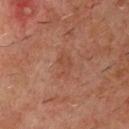Imaged during a routine full-body skin examination; the lesion was not biopsied and no histopathology is available. Automated image analysis of the tile measured a mean CIELAB color near L≈43 a*≈23 b*≈29, about 5 CIELAB-L* units darker than the surrounding skin, and a lesion-to-skin contrast of about 4.5 (normalized; higher = more distinct). The software also gave a color-variation rating of about 2/10. A male subject, in their 60s. The recorded lesion diameter is about 3.5 mm. This is a cross-polarized tile. On the chest. A 15 mm close-up extracted from a 3D total-body photography capture.